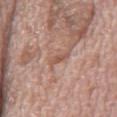Part of a total-body skin-imaging series; this lesion was reviewed on a skin check and was not flagged for biopsy.
Cropped from a total-body skin-imaging series; the visible field is about 15 mm.
The tile uses white-light illumination.
A male subject approximately 70 years of age.
The lesion is located on the mid back.